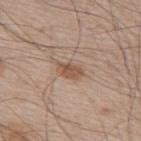Part of a total-body skin-imaging series; this lesion was reviewed on a skin check and was not flagged for biopsy. A male subject, aged 63–67. Imaged with white-light lighting. About 3 mm across. The total-body-photography lesion software estimated a lesion–skin lightness drop of about 10 and a normalized border contrast of about 7.5. It also reported an automated nevus-likeness rating near 65 out of 100. The lesion is located on the upper back. This image is a 15 mm lesion crop taken from a total-body photograph.